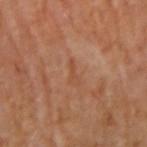The total-body-photography lesion software estimated an outline eccentricity of about 0.95 (0 = round, 1 = elongated). And it measured a lesion–skin lightness drop of about 6 and a normalized border contrast of about 5. And it measured a border-irregularity rating of about 6/10 and a within-lesion color-variation index near 0/10. The software also gave a classifier nevus-likeness of about 0/100 and a lesion-detection confidence of about 100/100. The recorded lesion diameter is about 3 mm. The tile uses cross-polarized illumination. From the right upper arm. A close-up tile cropped from a whole-body skin photograph, about 15 mm across.